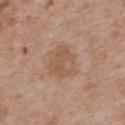Recorded during total-body skin imaging; not selected for excision or biopsy.
The lesion is located on the back.
The tile uses white-light illumination.
The patient is a female aged 28–32.
Cropped from a total-body skin-imaging series; the visible field is about 15 mm.